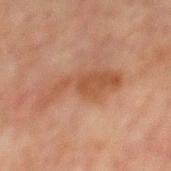Part of a total-body skin-imaging series; this lesion was reviewed on a skin check and was not flagged for biopsy. The recorded lesion diameter is about 7.5 mm. A male patient aged around 60. On the mid back. This image is a 15 mm lesion crop taken from a total-body photograph. Imaged with cross-polarized lighting.